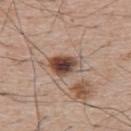biopsy status=catalogued during a skin exam; not biopsied
imaging modality=total-body-photography crop, ~15 mm field of view
patient=male, aged 63–67
illumination=white-light
site=the upper back